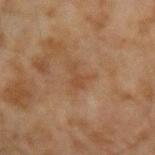follow-up: catalogued during a skin exam; not biopsied
location: the left forearm
tile lighting: cross-polarized illumination
lesion size: ≈2.5 mm
subject: male, aged around 45
image: ~15 mm crop, total-body skin-cancer survey